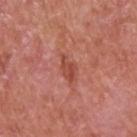Assessment: Recorded during total-body skin imaging; not selected for excision or biopsy. Clinical summary: The subject is a male roughly 65 years of age. The lesion is located on the back. About 3 mm across. A lesion tile, about 15 mm wide, cut from a 3D total-body photograph. The lesion-visualizer software estimated an eccentricity of roughly 0.8 and a symmetry-axis asymmetry near 0.25. The software also gave a border-irregularity index near 3/10, internal color variation of about 2.5 on a 0–10 scale, and peripheral color asymmetry of about 1. The tile uses white-light illumination.